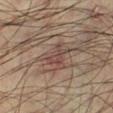workup = imaged on a skin check; not biopsied | image = ~15 mm tile from a whole-body skin photo | illumination = cross-polarized | image-analysis metrics = a classifier nevus-likeness of about 0/100 and lesion-presence confidence of about 80/100 | lesion diameter = ~4.5 mm (longest diameter) | location = the left lower leg | patient = male, aged around 35.